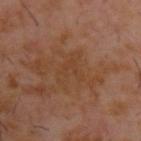biopsy status: total-body-photography surveillance lesion; no biopsy
lesion size: about 4.5 mm
automated metrics: a lesion area of about 8 mm², an outline eccentricity of about 0.8 (0 = round, 1 = elongated), and a shape-asymmetry score of about 0.45 (0 = symmetric); a mean CIELAB color near L≈37 a*≈19 b*≈30 and roughly 4 lightness units darker than nearby skin; a classifier nevus-likeness of about 0/100 and lesion-presence confidence of about 100/100
image source: total-body-photography crop, ~15 mm field of view
subject: male, approximately 60 years of age
body site: the upper back